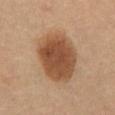No biopsy was performed on this lesion — it was imaged during a full skin examination and was not determined to be concerning.